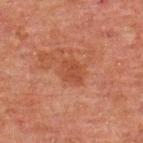- workup: no biopsy performed (imaged during a skin exam)
- anatomic site: the upper back
- subject: male, roughly 60 years of age
- size: about 3 mm
- imaging modality: ~15 mm tile from a whole-body skin photo
- image-analysis metrics: a lesion area of about 5 mm²; a lesion–skin lightness drop of about 5 and a lesion-to-skin contrast of about 5.5 (normalized; higher = more distinct); a border-irregularity rating of about 2/10, a within-lesion color-variation index near 1.5/10, and peripheral color asymmetry of about 0.5